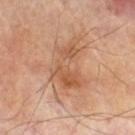Clinical summary: The total-body-photography lesion software estimated a footprint of about 12 mm² and two-axis asymmetry of about 0.5. This image is a 15 mm lesion crop taken from a total-body photograph. The tile uses cross-polarized illumination. The lesion's longest dimension is about 5.5 mm. A male patient, approximately 70 years of age. The lesion is on the right thigh.